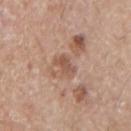Notes:
• biopsy status: total-body-photography surveillance lesion; no biopsy
• subject: male, aged 73 to 77
• acquisition: ~15 mm tile from a whole-body skin photo
• lighting: white-light
• location: the right upper arm
• automated lesion analysis: about 9 CIELAB-L* units darker than the surrounding skin and a lesion-to-skin contrast of about 6.5 (normalized; higher = more distinct)